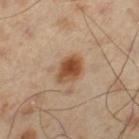– notes: catalogued during a skin exam; not biopsied
– subject: male, aged around 55
– anatomic site: the left thigh
– image source: ~15 mm crop, total-body skin-cancer survey